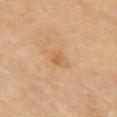Clinical impression: Captured during whole-body skin photography for melanoma surveillance; the lesion was not biopsied. Image and clinical context: Approximately 2.5 mm at its widest. A 15 mm crop from a total-body photograph taken for skin-cancer surveillance. The lesion is on the right upper arm.